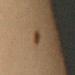biopsy status=no biopsy performed (imaged during a skin exam) | subject=female, aged 28–32 | location=the right upper arm | automated lesion analysis=a symmetry-axis asymmetry near 0.35; an average lesion color of about L≈37 a*≈14 b*≈28 (CIELAB), a lesion–skin lightness drop of about 9, and a normalized lesion–skin contrast near 8; a nevus-likeness score of about 100/100 and a lesion-detection confidence of about 100/100 | illumination=cross-polarized illumination | acquisition=total-body-photography crop, ~15 mm field of view.